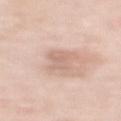illumination: white-light | image source: ~15 mm tile from a whole-body skin photo | subject: female, in their 50s | anatomic site: the upper back.